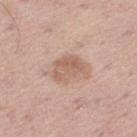notes — no biopsy performed (imaged during a skin exam) | acquisition — ~15 mm crop, total-body skin-cancer survey | anatomic site — the left thigh | subject — male, roughly 55 years of age | lighting — white-light.